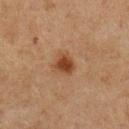Part of a total-body skin-imaging series; this lesion was reviewed on a skin check and was not flagged for biopsy. A male subject aged 73–77. The total-body-photography lesion software estimated an area of roughly 5 mm² and a symmetry-axis asymmetry near 0.25. And it measured a lesion color around L≈34 a*≈19 b*≈29 in CIELAB and roughly 10 lightness units darker than nearby skin. It also reported internal color variation of about 2 on a 0–10 scale and a peripheral color-asymmetry measure near 0.5. On the chest. A close-up tile cropped from a whole-body skin photograph, about 15 mm across. About 2.5 mm across.